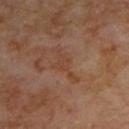The lesion was photographed on a routine skin check and not biopsied; there is no pathology result. Cropped from a whole-body photographic skin survey; the tile spans about 15 mm. Located on the upper back. The tile uses cross-polarized illumination. A male subject, in their 70s.